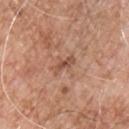The lesion was tiled from a total-body skin photograph and was not biopsied.
The subject is a male roughly 55 years of age.
The recorded lesion diameter is about 3 mm.
On the chest.
The tile uses white-light illumination.
This image is a 15 mm lesion crop taken from a total-body photograph.